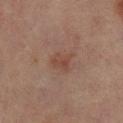follow-up: catalogued during a skin exam; not biopsied | lesion diameter: about 3 mm | body site: the leg | acquisition: ~15 mm tile from a whole-body skin photo | tile lighting: cross-polarized | subject: female, about 55 years old.